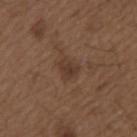body site = the left upper arm
automated lesion analysis = an average lesion color of about L≈36 a*≈17 b*≈25 (CIELAB) and roughly 7 lightness units darker than nearby skin; a border-irregularity index near 2.5/10, a color-variation rating of about 2/10, and radial color variation of about 0.5; a nevus-likeness score of about 45/100
acquisition = ~15 mm crop, total-body skin-cancer survey
patient = male, aged 48 to 52
illumination = white-light